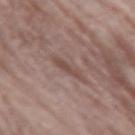This lesion was catalogued during total-body skin photography and was not selected for biopsy. Located on the left forearm. A female patient, aged 68–72. Approximately 3.5 mm at its widest. This image is a 15 mm lesion crop taken from a total-body photograph. The tile uses white-light illumination.